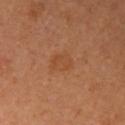{
  "biopsy_status": "not biopsied; imaged during a skin examination",
  "patient": {
    "sex": "female",
    "age_approx": 35
  },
  "image": {
    "source": "total-body photography crop",
    "field_of_view_mm": 15
  },
  "site": "right upper arm"
}